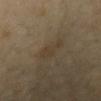Recorded during total-body skin imaging; not selected for excision or biopsy. Imaged with cross-polarized lighting. A close-up tile cropped from a whole-body skin photograph, about 15 mm across. The subject is a male about 65 years old. The lesion's longest dimension is about 3 mm. The lesion is located on the chest.